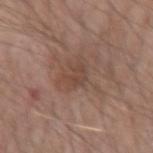biopsy_status: not biopsied; imaged during a skin examination
site: right forearm
patient:
  sex: male
  age_approx: 60
automated_metrics:
  vs_skin_darker_L: 6.0
  vs_skin_contrast_norm: 5.0
  border_irregularity_0_10: 3.5
  color_variation_0_10: 2.5
  peripheral_color_asymmetry: 1.0
  nevus_likeness_0_100: 0
  lesion_detection_confidence_0_100: 100
image:
  source: total-body photography crop
  field_of_view_mm: 15
lesion_size:
  long_diameter_mm_approx: 2.5